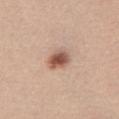follow-up: total-body-photography surveillance lesion; no biopsy | lesion diameter: ≈3 mm | body site: the chest | subject: female, aged 23–27 | automated metrics: an average lesion color of about L≈55 a*≈21 b*≈28 (CIELAB) and roughly 15 lightness units darker than nearby skin | acquisition: total-body-photography crop, ~15 mm field of view | tile lighting: white-light illumination.